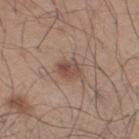Impression: Recorded during total-body skin imaging; not selected for excision or biopsy. Background: A male subject, aged around 45. A lesion tile, about 15 mm wide, cut from a 3D total-body photograph. Located on the right thigh.